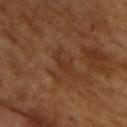Assessment: This lesion was catalogued during total-body skin photography and was not selected for biopsy. Context: An algorithmic analysis of the crop reported an area of roughly 3 mm², a shape eccentricity near 0.9, and two-axis asymmetry of about 0.5. It also reported a mean CIELAB color near L≈32 a*≈20 b*≈30 and roughly 5 lightness units darker than nearby skin. The analysis additionally found a classifier nevus-likeness of about 0/100 and a lesion-detection confidence of about 100/100. The recorded lesion diameter is about 2.5 mm. A male patient aged approximately 65. The tile uses cross-polarized illumination. A close-up tile cropped from a whole-body skin photograph, about 15 mm across.